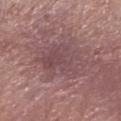Case summary:
* notes: imaged on a skin check; not biopsied
* diameter: ~6.5 mm (longest diameter)
* anatomic site: the left forearm
* subject: female, roughly 65 years of age
* acquisition: ~15 mm tile from a whole-body skin photo
* illumination: white-light